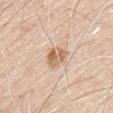Notes:
- follow-up: imaged on a skin check; not biopsied
- anatomic site: the upper back
- patient: male, approximately 70 years of age
- lesion diameter: about 4 mm
- image source: ~15 mm tile from a whole-body skin photo
- automated metrics: an area of roughly 6 mm² and two-axis asymmetry of about 0.35; border irregularity of about 3.5 on a 0–10 scale, internal color variation of about 5.5 on a 0–10 scale, and peripheral color asymmetry of about 2
- lighting: white-light illumination